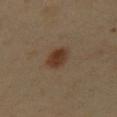Part of a total-body skin-imaging series; this lesion was reviewed on a skin check and was not flagged for biopsy. From the abdomen. The subject is a male about 50 years old. A 15 mm crop from a total-body photograph taken for skin-cancer surveillance.